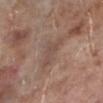notes: no biopsy performed (imaged during a skin exam) | automated metrics: a nevus-likeness score of about 0/100 and lesion-presence confidence of about 80/100 | acquisition: 15 mm crop, total-body photography | anatomic site: the left lower leg | illumination: white-light | subject: male, approximately 70 years of age | diameter: about 4 mm.